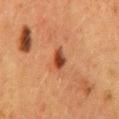This lesion was catalogued during total-body skin photography and was not selected for biopsy. Imaged with cross-polarized lighting. The lesion-visualizer software estimated a lesion area of about 4 mm². The software also gave an average lesion color of about L≈38 a*≈25 b*≈32 (CIELAB). And it measured border irregularity of about 2 on a 0–10 scale. A 15 mm close-up tile from a total-body photography series done for melanoma screening. The lesion's longest dimension is about 3 mm. A female patient roughly 50 years of age. Located on the mid back.